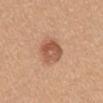follow-up: no biopsy performed (imaged during a skin exam)
illumination: white-light illumination
lesion size: ~4 mm (longest diameter)
location: the front of the torso
patient: female, approximately 55 years of age
image source: total-body-photography crop, ~15 mm field of view
TBP lesion metrics: a lesion area of about 9 mm²; an automated nevus-likeness rating near 75 out of 100 and a detector confidence of about 100 out of 100 that the crop contains a lesion